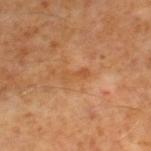Captured during whole-body skin photography for melanoma surveillance; the lesion was not biopsied. The subject is a male aged around 60. On the right lower leg. A 15 mm close-up extracted from a 3D total-body photography capture. The total-body-photography lesion software estimated a mean CIELAB color near L≈49 a*≈22 b*≈38, a lesion–skin lightness drop of about 6, and a lesion-to-skin contrast of about 5 (normalized; higher = more distinct).